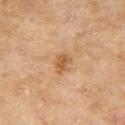<record>
<biopsy_status>not biopsied; imaged during a skin examination</biopsy_status>
<automated_metrics>
  <area_mm2_approx>4.0</area_mm2_approx>
  <eccentricity>0.75</eccentricity>
  <shape_asymmetry>0.3</shape_asymmetry>
  <nevus_likeness_0_100>20</nevus_likeness_0_100>
  <lesion_detection_confidence_0_100>100</lesion_detection_confidence_0_100>
</automated_metrics>
<lesion_size>
  <long_diameter_mm_approx>2.5</long_diameter_mm_approx>
</lesion_size>
<patient>
  <sex>female</sex>
  <age_approx>60</age_approx>
</patient>
<image>
  <source>total-body photography crop</source>
  <field_of_view_mm>15</field_of_view_mm>
</image>
<lighting>cross-polarized</lighting>
<site>right thigh</site>
</record>